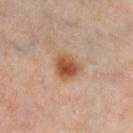Captured during whole-body skin photography for melanoma surveillance; the lesion was not biopsied. The tile uses cross-polarized illumination. The lesion's longest dimension is about 3 mm. A 15 mm close-up tile from a total-body photography series done for melanoma screening. The lesion is on the leg. A male subject approximately 55 years of age. The total-body-photography lesion software estimated peripheral color asymmetry of about 1.5.